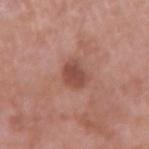follow-up: total-body-photography surveillance lesion; no biopsy | automated lesion analysis: a footprint of about 6 mm² and a shape eccentricity near 0.7; an average lesion color of about L≈48 a*≈25 b*≈26 (CIELAB), about 11 CIELAB-L* units darker than the surrounding skin, and a lesion-to-skin contrast of about 8 (normalized; higher = more distinct); an automated nevus-likeness rating near 50 out of 100 and a detector confidence of about 100 out of 100 that the crop contains a lesion | subject: male, aged 58–62 | size: ~3.5 mm (longest diameter) | illumination: white-light | acquisition: ~15 mm tile from a whole-body skin photo | site: the arm.